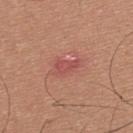notes: imaged on a skin check; not biopsied
illumination: white-light illumination
patient: male, aged 33–37
lesion diameter: ~3 mm (longest diameter)
image source: total-body-photography crop, ~15 mm field of view
location: the upper back